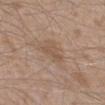workup = catalogued during a skin exam; not biopsied
imaging modality = 15 mm crop, total-body photography
diameter = ~3 mm (longest diameter)
illumination = white-light illumination
anatomic site = the right lower leg
automated lesion analysis = a footprint of about 4.5 mm² and a symmetry-axis asymmetry near 0.45; a classifier nevus-likeness of about 0/100 and a lesion-detection confidence of about 100/100
subject = male, aged approximately 45